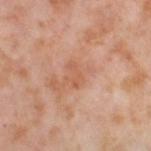{"biopsy_status": "not biopsied; imaged during a skin examination", "lesion_size": {"long_diameter_mm_approx": 3.0}, "patient": {"sex": "female", "age_approx": 55}, "site": "left thigh", "image": {"source": "total-body photography crop", "field_of_view_mm": 15}}